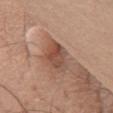<case>
<biopsy_status>not biopsied; imaged during a skin examination</biopsy_status>
<image>
  <source>total-body photography crop</source>
  <field_of_view_mm>15</field_of_view_mm>
</image>
<lighting>white-light</lighting>
<lesion_size>
  <long_diameter_mm_approx>4.5</long_diameter_mm_approx>
</lesion_size>
<patient>
  <sex>male</sex>
  <age_approx>75</age_approx>
</patient>
<site>chest</site>
</case>